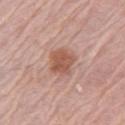Imaged during a routine full-body skin examination; the lesion was not biopsied and no histopathology is available.
From the arm.
A female subject, in their mid-60s.
Captured under white-light illumination.
A 15 mm close-up extracted from a 3D total-body photography capture.